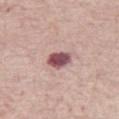A 15 mm crop from a total-body photograph taken for skin-cancer surveillance.
The lesion is on the right thigh.
Automated image analysis of the tile measured a mean CIELAB color near L≈51 a*≈25 b*≈16, a lesion–skin lightness drop of about 18, and a normalized lesion–skin contrast near 12.5. The software also gave a border-irregularity index near 1.5/10 and a color-variation rating of about 6/10.
A female patient, aged approximately 70.
About 3 mm across.
The tile uses white-light illumination.